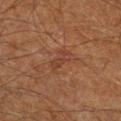biopsy status: catalogued during a skin exam; not biopsied
diameter: ≈3 mm
image: ~15 mm crop, total-body skin-cancer survey
patient: male, aged 58–62
tile lighting: cross-polarized
anatomic site: the right lower leg
image-analysis metrics: an area of roughly 3.5 mm² and a shape-asymmetry score of about 0.35 (0 = symmetric); an average lesion color of about L≈30 a*≈19 b*≈24 (CIELAB), a lesion–skin lightness drop of about 5, and a normalized border contrast of about 5; internal color variation of about 0 on a 0–10 scale; a classifier nevus-likeness of about 0/100